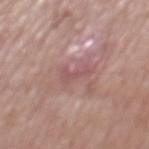Captured during whole-body skin photography for melanoma surveillance; the lesion was not biopsied. A 15 mm crop from a total-body photograph taken for skin-cancer surveillance. Captured under white-light illumination. On the mid back. Measured at roughly 3 mm in maximum diameter. The subject is a male in their mid-60s.